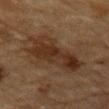Notes:
- workup · no biopsy performed (imaged during a skin exam)
- patient · male, approximately 85 years of age or older
- body site · the mid back
- lesion diameter · about 8.5 mm
- illumination · cross-polarized
- image · ~15 mm tile from a whole-body skin photo